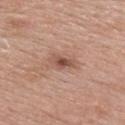Case summary:
- notes: no biopsy performed (imaged during a skin exam)
- body site: the upper back
- tile lighting: white-light illumination
- subject: female, roughly 50 years of age
- image: 15 mm crop, total-body photography
- lesion size: ~3 mm (longest diameter)